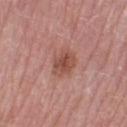Case summary:
- follow-up: catalogued during a skin exam; not biopsied
- body site: the right lower leg
- image: ~15 mm tile from a whole-body skin photo
- lesion size: ≈3 mm
- image-analysis metrics: a border-irregularity index near 1.5/10 and peripheral color asymmetry of about 1; a nevus-likeness score of about 65/100 and lesion-presence confidence of about 100/100
- patient: female, roughly 70 years of age
- illumination: white-light illumination